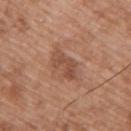Notes:
• notes · imaged on a skin check; not biopsied
• tile lighting · white-light
• patient · male, in their 50s
• automated metrics · a lesion area of about 6.5 mm²; an average lesion color of about L≈49 a*≈22 b*≈30 (CIELAB) and a normalized border contrast of about 6.5
• lesion size · ≈4 mm
• body site · the upper back
• image source · 15 mm crop, total-body photography